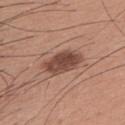| feature | finding |
|---|---|
| notes | catalogued during a skin exam; not biopsied |
| lighting | white-light |
| image source | 15 mm crop, total-body photography |
| site | the upper back |
| patient | male, in their mid- to late 30s |